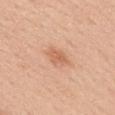follow-up: total-body-photography surveillance lesion; no biopsy
site: the upper back
lighting: white-light
imaging modality: ~15 mm crop, total-body skin-cancer survey
TBP lesion metrics: a footprint of about 5 mm², an outline eccentricity of about 0.75 (0 = round, 1 = elongated), and a shape-asymmetry score of about 0.2 (0 = symmetric); a border-irregularity rating of about 2.5/10, internal color variation of about 2 on a 0–10 scale, and radial color variation of about 0.5; an automated nevus-likeness rating near 65 out of 100 and lesion-presence confidence of about 100/100
lesion size: ≈3 mm
subject: female, aged 38–42